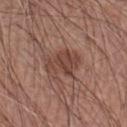{"biopsy_status": "not biopsied; imaged during a skin examination", "patient": {"sex": "male", "age_approx": 60}, "lesion_size": {"long_diameter_mm_approx": 4.5}, "image": {"source": "total-body photography crop", "field_of_view_mm": 15}, "site": "left forearm", "lighting": "white-light"}